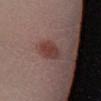The lesion is on the leg. This is a cross-polarized tile. About 4 mm across. A female subject, aged 38 to 42. Cropped from a whole-body photographic skin survey; the tile spans about 15 mm.